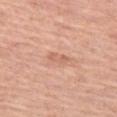Image and clinical context: Cropped from a whole-body photographic skin survey; the tile spans about 15 mm. Located on the left thigh. A male subject in their mid-70s. Longest diameter approximately 3 mm. The tile uses white-light illumination.